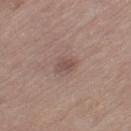This image is a 15 mm lesion crop taken from a total-body photograph.
Longest diameter approximately 2.5 mm.
The lesion is located on the left thigh.
A female patient aged 63–67.
The total-body-photography lesion software estimated a mean CIELAB color near L≈49 a*≈18 b*≈21 and a normalized border contrast of about 6. It also reported border irregularity of about 2 on a 0–10 scale, a color-variation rating of about 1.5/10, and radial color variation of about 0.5.